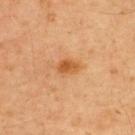Assessment:
No biopsy was performed on this lesion — it was imaged during a full skin examination and was not determined to be concerning.
Acquisition and patient details:
An algorithmic analysis of the crop reported a border-irregularity index near 2.5/10, internal color variation of about 3.5 on a 0–10 scale, and peripheral color asymmetry of about 1. It also reported an automated nevus-likeness rating near 65 out of 100 and lesion-presence confidence of about 100/100. From the upper back. About 3 mm across. A close-up tile cropped from a whole-body skin photograph, about 15 mm across. This is a cross-polarized tile. A male patient, aged 48–52.